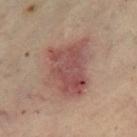* notes: catalogued during a skin exam; not biopsied
* location: the right thigh
* lesion diameter: ≈7 mm
* patient: female, aged approximately 60
* tile lighting: cross-polarized illumination
* image source: total-body-photography crop, ~15 mm field of view
* automated lesion analysis: about 10 CIELAB-L* units darker than the surrounding skin and a normalized lesion–skin contrast near 8; a border-irregularity index near 3.5/10, internal color variation of about 5.5 on a 0–10 scale, and a peripheral color-asymmetry measure near 2